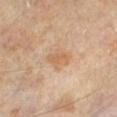The lesion was tiled from a total-body skin photograph and was not biopsied. The patient is a male roughly 65 years of age. On the leg. A 15 mm close-up extracted from a 3D total-body photography capture. The lesion's longest dimension is about 3.5 mm. Captured under cross-polarized illumination.